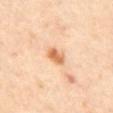| key | value |
|---|---|
| follow-up | imaged on a skin check; not biopsied |
| image | total-body-photography crop, ~15 mm field of view |
| lesion size | about 3 mm |
| subject | female, aged approximately 60 |
| illumination | cross-polarized illumination |
| anatomic site | the mid back |
| automated metrics | an area of roughly 4 mm², an eccentricity of roughly 0.8, and two-axis asymmetry of about 0.25; a mean CIELAB color near L≈68 a*≈24 b*≈41 and a normalized lesion–skin contrast near 9; border irregularity of about 2.5 on a 0–10 scale, a within-lesion color-variation index near 4/10, and radial color variation of about 1.5; an automated nevus-likeness rating near 95 out of 100 and a detector confidence of about 100 out of 100 that the crop contains a lesion |